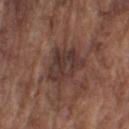lesion diameter: about 5 mm
subject: male, aged approximately 75
body site: the arm
automated metrics: a lesion area of about 12 mm² and a shape-asymmetry score of about 0.3 (0 = symmetric); border irregularity of about 4 on a 0–10 scale and peripheral color asymmetry of about 1.5
tile lighting: white-light
acquisition: total-body-photography crop, ~15 mm field of view